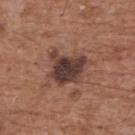biopsy_status: not biopsied; imaged during a skin examination